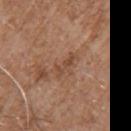workup = total-body-photography surveillance lesion; no biopsy
image = total-body-photography crop, ~15 mm field of view
subject = male, aged 58–62
lesion diameter = ≈3 mm
image-analysis metrics = a mean CIELAB color near L≈47 a*≈20 b*≈30, about 7 CIELAB-L* units darker than the surrounding skin, and a normalized border contrast of about 6
site = the arm
tile lighting = white-light illumination